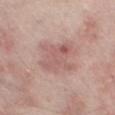follow-up: imaged on a skin check; not biopsied | subject: male, in their mid-60s | TBP lesion metrics: an eccentricity of roughly 0.5 and a symmetry-axis asymmetry near 0.3; an average lesion color of about L≈58 a*≈22 b*≈23 (CIELAB), about 8 CIELAB-L* units darker than the surrounding skin, and a normalized border contrast of about 5.5 | imaging modality: ~15 mm crop, total-body skin-cancer survey | tile lighting: white-light | anatomic site: the leg | diameter: ~4.5 mm (longest diameter).